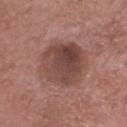{
  "biopsy_status": "not biopsied; imaged during a skin examination",
  "lighting": "white-light",
  "site": "head or neck",
  "image": {
    "source": "total-body photography crop",
    "field_of_view_mm": 15
  },
  "automated_metrics": {
    "area_mm2_approx": 21.0,
    "eccentricity": 0.55,
    "shape_asymmetry": 0.15,
    "cielab_L": 44,
    "cielab_a": 21,
    "cielab_b": 23,
    "vs_skin_darker_L": 11.0,
    "vs_skin_contrast_norm": 8.0
  },
  "patient": {
    "sex": "male",
    "age_approx": 75
  }
}